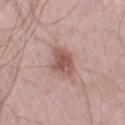Q: Was a biopsy performed?
A: catalogued during a skin exam; not biopsied
Q: How was this image acquired?
A: 15 mm crop, total-body photography
Q: Illumination type?
A: white-light illumination
Q: What did automated image analysis measure?
A: a lesion area of about 8.5 mm², an outline eccentricity of about 0.8 (0 = round, 1 = elongated), and a symmetry-axis asymmetry near 0.25; an automated nevus-likeness rating near 80 out of 100
Q: Patient demographics?
A: male, approximately 55 years of age
Q: Where on the body is the lesion?
A: the leg
Q: What is the lesion's diameter?
A: about 4.5 mm